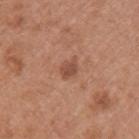Part of a total-body skin-imaging series; this lesion was reviewed on a skin check and was not flagged for biopsy. The lesion's longest dimension is about 2.5 mm. A roughly 15 mm field-of-view crop from a total-body skin photograph. A female subject in their 40s. Located on the left upper arm. This is a white-light tile.